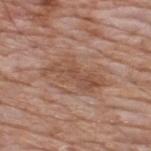This lesion was catalogued during total-body skin photography and was not selected for biopsy. A male subject aged 58 to 62. This is a white-light tile. On the upper back. A lesion tile, about 15 mm wide, cut from a 3D total-body photograph. Automated image analysis of the tile measured border irregularity of about 6 on a 0–10 scale, a within-lesion color-variation index near 3.5/10, and radial color variation of about 1.5.